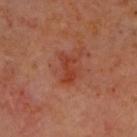Imaged during a routine full-body skin examination; the lesion was not biopsied and no histopathology is available.